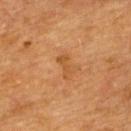Clinical impression:
Recorded during total-body skin imaging; not selected for excision or biopsy.
Acquisition and patient details:
A female patient, in their mid-50s. Located on the upper back. Approximately 3 mm at its widest. A close-up tile cropped from a whole-body skin photograph, about 15 mm across. Imaged with cross-polarized lighting.